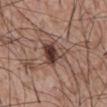Findings:
– notes: catalogued during a skin exam; not biopsied
– patient: male, roughly 60 years of age
– body site: the abdomen
– image: ~15 mm crop, total-body skin-cancer survey
– tile lighting: white-light
– lesion size: ~3.5 mm (longest diameter)